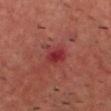Recorded during total-body skin imaging; not selected for excision or biopsy.
The subject is a male aged 43 to 47.
A region of skin cropped from a whole-body photographic capture, roughly 15 mm wide.
This is a cross-polarized tile.
Located on the head or neck.
An algorithmic analysis of the crop reported an area of roughly 6 mm² and a shape eccentricity near 0.55. And it measured roughly 8 lightness units darker than nearby skin and a normalized lesion–skin contrast near 7. And it measured border irregularity of about 3 on a 0–10 scale, a within-lesion color-variation index near 4/10, and peripheral color asymmetry of about 1.5. It also reported a detector confidence of about 100 out of 100 that the crop contains a lesion.
About 3 mm across.